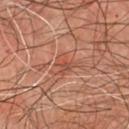Recorded during total-body skin imaging; not selected for excision or biopsy. From the chest. A close-up tile cropped from a whole-body skin photograph, about 15 mm across. The patient is a male aged 63–67. Imaged with cross-polarized lighting. The recorded lesion diameter is about 2.5 mm.